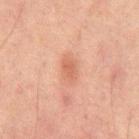This lesion was catalogued during total-body skin photography and was not selected for biopsy.
On the mid back.
This image is a 15 mm lesion crop taken from a total-body photograph.
Captured under cross-polarized illumination.
A male patient aged around 65.
The total-body-photography lesion software estimated a detector confidence of about 100 out of 100 that the crop contains a lesion.
The lesion's longest dimension is about 3 mm.